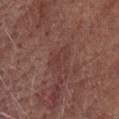The lesion was tiled from a total-body skin photograph and was not biopsied.
Longest diameter approximately 3 mm.
This image is a 15 mm lesion crop taken from a total-body photograph.
Located on the head or neck.
A female subject, aged approximately 75.
Imaged with white-light lighting.